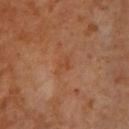notes = imaged on a skin check; not biopsied | diameter = ≈3 mm | image source = total-body-photography crop, ~15 mm field of view | location = the chest | illumination = cross-polarized | subject = female | TBP lesion metrics = an average lesion color of about L≈48 a*≈25 b*≈36 (CIELAB), a lesion–skin lightness drop of about 5, and a normalized border contrast of about 5; an automated nevus-likeness rating near 0 out of 100 and a lesion-detection confidence of about 100/100.